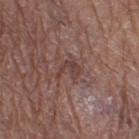Assessment: The lesion was tiled from a total-body skin photograph and was not biopsied. Context: A lesion tile, about 15 mm wide, cut from a 3D total-body photograph. Located on the right thigh. A male patient in their mid-60s.